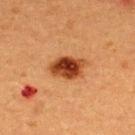Clinical impression: Recorded during total-body skin imaging; not selected for excision or biopsy. Acquisition and patient details: The subject is a male approximately 40 years of age. Cropped from a total-body skin-imaging series; the visible field is about 15 mm. On the upper back. Imaged with cross-polarized lighting. About 4.5 mm across.